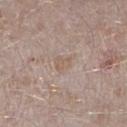Q: Was a biopsy performed?
A: catalogued during a skin exam; not biopsied
Q: How was this image acquired?
A: ~15 mm crop, total-body skin-cancer survey
Q: Where on the body is the lesion?
A: the left lower leg
Q: Automated lesion metrics?
A: a footprint of about 4 mm², an eccentricity of roughly 0.65, and a symmetry-axis asymmetry near 0.35; a lesion color around L≈58 a*≈14 b*≈25 in CIELAB and a lesion–skin lightness drop of about 5; border irregularity of about 3.5 on a 0–10 scale and peripheral color asymmetry of about 0.5; an automated nevus-likeness rating near 0 out of 100 and a detector confidence of about 95 out of 100 that the crop contains a lesion
Q: Who is the patient?
A: male, approximately 45 years of age
Q: What is the lesion's diameter?
A: about 2.5 mm
Q: How was the tile lit?
A: white-light